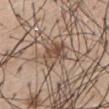Clinical impression: This lesion was catalogued during total-body skin photography and was not selected for biopsy. Context: Located on the back. The recorded lesion diameter is about 5 mm. A male patient in their mid-50s. Cropped from a whole-body photographic skin survey; the tile spans about 15 mm. The tile uses white-light illumination.